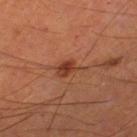Context: Captured under cross-polarized illumination. Longest diameter approximately 3.5 mm. The lesion-visualizer software estimated roughly 8 lightness units darker than nearby skin. And it measured border irregularity of about 5 on a 0–10 scale, internal color variation of about 4 on a 0–10 scale, and radial color variation of about 1.5. The analysis additionally found a nevus-likeness score of about 95/100 and lesion-presence confidence of about 100/100. A lesion tile, about 15 mm wide, cut from a 3D total-body photograph. On the left thigh. The patient is a male in their mid- to late 60s.